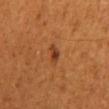Image and clinical context:
Automated image analysis of the tile measured a lesion color around L≈38 a*≈25 b*≈35 in CIELAB, about 9 CIELAB-L* units darker than the surrounding skin, and a normalized border contrast of about 8. And it measured a border-irregularity index near 3/10 and internal color variation of about 2.5 on a 0–10 scale. The lesion is located on the mid back. Approximately 2.5 mm at its widest. A 15 mm close-up tile from a total-body photography series done for melanoma screening. The patient is a male about 45 years old.